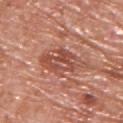Clinical impression: The lesion was photographed on a routine skin check and not biopsied; there is no pathology result. Context: Automated image analysis of the tile measured a shape eccentricity near 0.85 and a shape-asymmetry score of about 0.25 (0 = symmetric). The software also gave a nevus-likeness score of about 0/100 and a detector confidence of about 80 out of 100 that the crop contains a lesion. The lesion's longest dimension is about 6 mm. A male patient aged 63 to 67. On the upper back. A 15 mm close-up extracted from a 3D total-body photography capture.